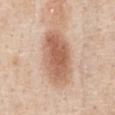<record>
  <biopsy_status>not biopsied; imaged during a skin examination</biopsy_status>
  <automated_metrics>
    <border_irregularity_0_10>1.5</border_irregularity_0_10>
    <color_variation_0_10>4.5</color_variation_0_10>
    <peripheral_color_asymmetry>1.5</peripheral_color_asymmetry>
    <nevus_likeness_0_100>100</nevus_likeness_0_100>
    <lesion_detection_confidence_0_100>100</lesion_detection_confidence_0_100>
  </automated_metrics>
  <lesion_size>
    <long_diameter_mm_approx>6.5</long_diameter_mm_approx>
  </lesion_size>
  <patient>
    <sex>male</sex>
    <age_approx>60</age_approx>
  </patient>
  <image>
    <source>total-body photography crop</source>
    <field_of_view_mm>15</field_of_view_mm>
  </image>
  <lighting>white-light</lighting>
  <site>chest</site>
</record>